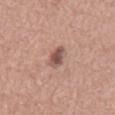| feature | finding |
|---|---|
| notes | no biopsy performed (imaged during a skin exam) |
| imaging modality | ~15 mm crop, total-body skin-cancer survey |
| subject | male, roughly 65 years of age |
| body site | the mid back |